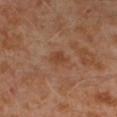Findings:
* anatomic site: the left lower leg
* image source: ~15 mm crop, total-body skin-cancer survey
* size: about 2.5 mm
* patient: male, aged 28 to 32